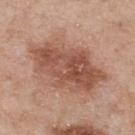follow-up — no biopsy performed (imaged during a skin exam) | subject — male, aged 53–57 | tile lighting — white-light | acquisition — ~15 mm crop, total-body skin-cancer survey | location — the upper back | diameter — about 9.5 mm.